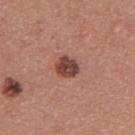Q: Is there a histopathology result?
A: catalogued during a skin exam; not biopsied
Q: Patient demographics?
A: male, in their 40s
Q: Where on the body is the lesion?
A: the back
Q: How was this image acquired?
A: 15 mm crop, total-body photography